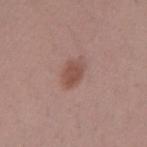Impression: This lesion was catalogued during total-body skin photography and was not selected for biopsy. Background: A male subject, approximately 35 years of age. Located on the right forearm. The recorded lesion diameter is about 3 mm. An algorithmic analysis of the crop reported a footprint of about 5.5 mm² and an outline eccentricity of about 0.6 (0 = round, 1 = elongated). And it measured border irregularity of about 2 on a 0–10 scale, a color-variation rating of about 2/10, and radial color variation of about 0.5. Cropped from a whole-body photographic skin survey; the tile spans about 15 mm.